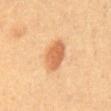{
  "biopsy_status": "not biopsied; imaged during a skin examination",
  "site": "abdomen",
  "image": {
    "source": "total-body photography crop",
    "field_of_view_mm": 15
  },
  "patient": {
    "sex": "male",
    "age_approx": 60
  }
}